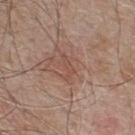From the upper back. This image is a 15 mm lesion crop taken from a total-body photograph. The subject is a male in their mid-50s. The total-body-photography lesion software estimated an average lesion color of about L≈49 a*≈20 b*≈25 (CIELAB), a lesion–skin lightness drop of about 6, and a normalized border contrast of about 5. It also reported an automated nevus-likeness rating near 0 out of 100 and a lesion-detection confidence of about 90/100. This is a white-light tile.